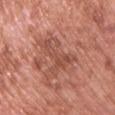Q: What is the anatomic site?
A: the front of the torso
Q: What is the imaging modality?
A: total-body-photography crop, ~15 mm field of view
Q: What is the lesion's diameter?
A: ≈6 mm
Q: What are the patient's age and sex?
A: male, roughly 70 years of age
Q: How was the tile lit?
A: white-light illumination